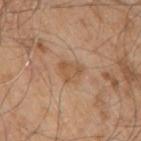* notes: imaged on a skin check; not biopsied
* subject: male, roughly 80 years of age
* site: the right upper arm
* lesion diameter: ~2.5 mm (longest diameter)
* image source: total-body-photography crop, ~15 mm field of view
* tile lighting: white-light